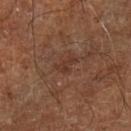| feature | finding |
|---|---|
| biopsy status | catalogued during a skin exam; not biopsied |
| lesion size | ~2.5 mm (longest diameter) |
| tile lighting | cross-polarized illumination |
| acquisition | total-body-photography crop, ~15 mm field of view |
| subject | aged 63–67 |
| location | the leg |
| automated lesion analysis | an area of roughly 3 mm², an eccentricity of roughly 0.8, and a symmetry-axis asymmetry near 0.55; a lesion color around L≈34 a*≈20 b*≈26 in CIELAB and about 6 CIELAB-L* units darker than the surrounding skin; a border-irregularity rating of about 6/10, a within-lesion color-variation index near 0/10, and a peripheral color-asymmetry measure near 0 |